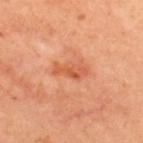workup — catalogued during a skin exam; not biopsied
automated metrics — an area of roughly 5 mm² and a shape eccentricity near 0.9
subject — female, approximately 45 years of age
image source — ~15 mm tile from a whole-body skin photo
illumination — cross-polarized illumination
lesion diameter — about 4 mm
body site — the back